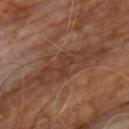  biopsy_status: not biopsied; imaged during a skin examination
  site: chest
  patient:
    sex: male
    age_approx: 60
  lighting: cross-polarized
  image:
    source: total-body photography crop
    field_of_view_mm: 15
  lesion_size:
    long_diameter_mm_approx: 3.0